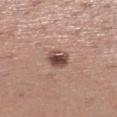Context: The lesion is on the leg. A 15 mm close-up extracted from a 3D total-body photography capture. The tile uses white-light illumination. About 2.5 mm across. A female patient aged around 30.